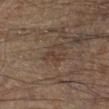Recorded during total-body skin imaging; not selected for excision or biopsy. The subject is a male about 65 years old. A close-up tile cropped from a whole-body skin photograph, about 15 mm across. On the right lower leg. The recorded lesion diameter is about 3 mm.